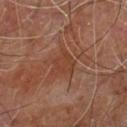lighting: cross-polarized; site: the leg; size: ≈3 mm; patient: male, approximately 60 years of age; acquisition: ~15 mm crop, total-body skin-cancer survey.